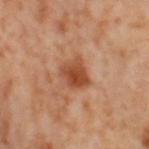On the left thigh.
Automated image analysis of the tile measured a mean CIELAB color near L≈49 a*≈27 b*≈36, roughly 12 lightness units darker than nearby skin, and a normalized lesion–skin contrast near 9. The software also gave a nevus-likeness score of about 95/100 and lesion-presence confidence of about 100/100.
Captured under cross-polarized illumination.
Longest diameter approximately 3.5 mm.
A female subject aged approximately 55.
A lesion tile, about 15 mm wide, cut from a 3D total-body photograph.